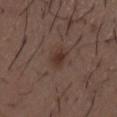Notes:
• biopsy status: total-body-photography surveillance lesion; no biopsy
• subject: male, in their 50s
• imaging modality: 15 mm crop, total-body photography
• body site: the back